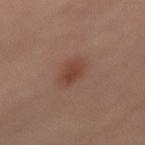Part of a total-body skin-imaging series; this lesion was reviewed on a skin check and was not flagged for biopsy. A close-up tile cropped from a whole-body skin photograph, about 15 mm across. Captured under cross-polarized illumination. Located on the left thigh. About 4 mm across. A female subject in their mid-40s. An algorithmic analysis of the crop reported roughly 8 lightness units darker than nearby skin.